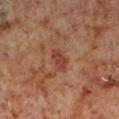<case>
<biopsy_status>not biopsied; imaged during a skin examination</biopsy_status>
<patient>
  <sex>male</sex>
  <age_approx>60</age_approx>
</patient>
<site>left lower leg</site>
<lighting>cross-polarized</lighting>
<image>
  <source>total-body photography crop</source>
  <field_of_view_mm>15</field_of_view_mm>
</image>
<lesion_size>
  <long_diameter_mm_approx>3.0</long_diameter_mm_approx>
</lesion_size>
</case>